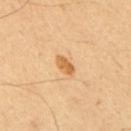Q: Was this lesion biopsied?
A: catalogued during a skin exam; not biopsied
Q: How was this image acquired?
A: ~15 mm tile from a whole-body skin photo
Q: Where on the body is the lesion?
A: the mid back
Q: Illumination type?
A: cross-polarized
Q: Patient demographics?
A: male, in their mid- to late 60s
Q: Lesion size?
A: ≈3 mm